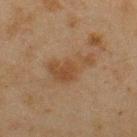- workup: imaged on a skin check; not biopsied
- subject: male, aged 43–47
- image: ~15 mm crop, total-body skin-cancer survey
- tile lighting: cross-polarized illumination
- lesion diameter: ~5.5 mm (longest diameter)
- anatomic site: the upper back